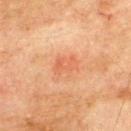This lesion was catalogued during total-body skin photography and was not selected for biopsy. A roughly 15 mm field-of-view crop from a total-body skin photograph. Imaged with cross-polarized lighting. The lesion is on the back. A male patient in their mid- to late 70s. The total-body-photography lesion software estimated an average lesion color of about L≈52 a*≈25 b*≈34 (CIELAB) and a lesion–skin lightness drop of about 6. It also reported a classifier nevus-likeness of about 0/100. Measured at roughly 2.5 mm in maximum diameter.